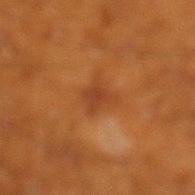Impression:
No biopsy was performed on this lesion — it was imaged during a full skin examination and was not determined to be concerning.
Acquisition and patient details:
Cropped from a total-body skin-imaging series; the visible field is about 15 mm. On the left lower leg. The patient is a male in their 60s. Captured under cross-polarized illumination. Longest diameter approximately 3 mm.